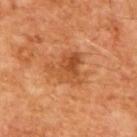The lesion was photographed on a routine skin check and not biopsied; there is no pathology result. Cropped from a total-body skin-imaging series; the visible field is about 15 mm. The total-body-photography lesion software estimated a lesion area of about 10 mm² and a shape-asymmetry score of about 0.5 (0 = symmetric). And it measured a lesion color around L≈50 a*≈28 b*≈41 in CIELAB and a normalized border contrast of about 6.5. It also reported border irregularity of about 5.5 on a 0–10 scale, a color-variation rating of about 5.5/10, and peripheral color asymmetry of about 2. Imaged with cross-polarized lighting. A male subject approximately 65 years of age.